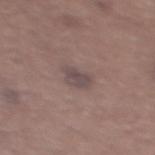notes — imaged on a skin check; not biopsied
anatomic site — the back
automated lesion analysis — an average lesion color of about L≈46 a*≈14 b*≈15 (CIELAB) and a normalized lesion–skin contrast near 7; border irregularity of about 3 on a 0–10 scale, a color-variation rating of about 2/10, and a peripheral color-asymmetry measure near 1
acquisition — total-body-photography crop, ~15 mm field of view
subject — female, roughly 65 years of age
illumination — white-light
lesion diameter — ≈3.5 mm